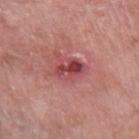notes: catalogued during a skin exam; not biopsied
TBP lesion metrics: a detector confidence of about 100 out of 100 that the crop contains a lesion
acquisition: 15 mm crop, total-body photography
illumination: white-light illumination
patient: female, aged 58–62
diameter: ≈4 mm
location: the left forearm